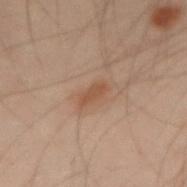{"image": {"source": "total-body photography crop", "field_of_view_mm": 15}, "patient": {"sex": "male", "age_approx": 50}, "lesion_size": {"long_diameter_mm_approx": 2.5}, "lighting": "cross-polarized", "site": "left arm"}